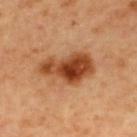Captured during whole-body skin photography for melanoma surveillance; the lesion was not biopsied. An algorithmic analysis of the crop reported a lesion color around L≈45 a*≈26 b*≈37 in CIELAB and a lesion-to-skin contrast of about 11 (normalized; higher = more distinct). The analysis additionally found border irregularity of about 3 on a 0–10 scale, internal color variation of about 8 on a 0–10 scale, and radial color variation of about 2.5. A male subject roughly 60 years of age. The tile uses cross-polarized illumination. From the upper back. About 6.5 mm across. Cropped from a total-body skin-imaging series; the visible field is about 15 mm.